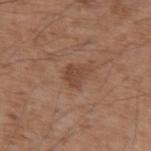follow-up = total-body-photography surveillance lesion; no biopsy | lesion size = ~3 mm (longest diameter) | illumination = white-light | site = the left upper arm | subject = male, approximately 55 years of age | acquisition = ~15 mm tile from a whole-body skin photo.